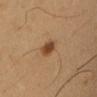This lesion was catalogued during total-body skin photography and was not selected for biopsy.
Cropped from a whole-body photographic skin survey; the tile spans about 15 mm.
The patient is a male in their 50s.
Measured at roughly 2.5 mm in maximum diameter.
Located on the right upper arm.